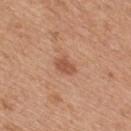biopsy_status: not biopsied; imaged during a skin examination
site: upper back
patient:
  sex: female
  age_approx: 45
lesion_size:
  long_diameter_mm_approx: 2.5
image:
  source: total-body photography crop
  field_of_view_mm: 15